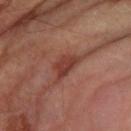Recorded during total-body skin imaging; not selected for excision or biopsy. Captured under cross-polarized illumination. The total-body-photography lesion software estimated an area of roughly 5 mm², an outline eccentricity of about 0.8 (0 = round, 1 = elongated), and two-axis asymmetry of about 0.35. It also reported a border-irregularity rating of about 3.5/10, internal color variation of about 2.5 on a 0–10 scale, and peripheral color asymmetry of about 1. And it measured an automated nevus-likeness rating near 25 out of 100. Cropped from a whole-body photographic skin survey; the tile spans about 15 mm. The lesion is located on the left forearm. The patient is a male roughly 85 years of age or older. Longest diameter approximately 3 mm.